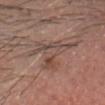This lesion was catalogued during total-body skin photography and was not selected for biopsy.
The lesion is located on the head or neck.
A region of skin cropped from a whole-body photographic capture, roughly 15 mm wide.
The patient is a male approximately 65 years of age.
Measured at roughly 7.5 mm in maximum diameter.
Captured under cross-polarized illumination.
The total-body-photography lesion software estimated two-axis asymmetry of about 0.7. And it measured a nevus-likeness score of about 0/100 and lesion-presence confidence of about 65/100.